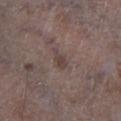biopsy status = imaged on a skin check; not biopsied | patient = male, about 70 years old | imaging modality = ~15 mm tile from a whole-body skin photo | lesion diameter = ~2.5 mm (longest diameter) | tile lighting = white-light illumination | body site = the left lower leg.